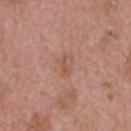| key | value |
|---|---|
| biopsy status | no biopsy performed (imaged during a skin exam) |
| subject | male, about 75 years old |
| anatomic site | the head or neck |
| lighting | white-light |
| imaging modality | 15 mm crop, total-body photography |
| lesion size | ≈2.5 mm |